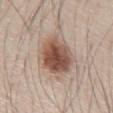Captured during whole-body skin photography for melanoma surveillance; the lesion was not biopsied. Captured under white-light illumination. The lesion's longest dimension is about 6 mm. The lesion is located on the abdomen. A close-up tile cropped from a whole-body skin photograph, about 15 mm across. Automated image analysis of the tile measured a footprint of about 19 mm², an outline eccentricity of about 0.75 (0 = round, 1 = elongated), and a shape-asymmetry score of about 0.15 (0 = symmetric). The analysis additionally found an automated nevus-likeness rating near 100 out of 100 and a lesion-detection confidence of about 100/100. A male patient, in their mid-40s.